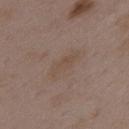Imaged during a routine full-body skin examination; the lesion was not biopsied and no histopathology is available. Captured under white-light illumination. Located on the upper back. The total-body-photography lesion software estimated a footprint of about 5 mm² and a symmetry-axis asymmetry near 0.35. And it measured a lesion color around L≈48 a*≈14 b*≈25 in CIELAB, a lesion–skin lightness drop of about 5, and a normalized border contrast of about 5. Cropped from a total-body skin-imaging series; the visible field is about 15 mm. Approximately 4 mm at its widest. A female patient, aged approximately 35.